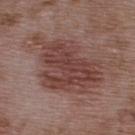Part of a total-body skin-imaging series; this lesion was reviewed on a skin check and was not flagged for biopsy. The total-body-photography lesion software estimated a lesion–skin lightness drop of about 10 and a lesion-to-skin contrast of about 8 (normalized; higher = more distinct). The software also gave a border-irregularity index near 5/10 and a peripheral color-asymmetry measure near 1.5. Measured at roughly 9 mm in maximum diameter. From the upper back. Captured under white-light illumination. The subject is a male in their 50s. This image is a 15 mm lesion crop taken from a total-body photograph.